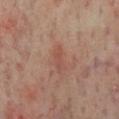Q: Was a biopsy performed?
A: no biopsy performed (imaged during a skin exam)
Q: Patient demographics?
A: male, roughly 65 years of age
Q: What is the imaging modality?
A: 15 mm crop, total-body photography
Q: What is the anatomic site?
A: the chest